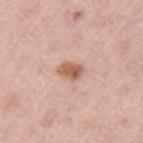Notes:
- follow-up · total-body-photography surveillance lesion; no biopsy
- lighting · white-light illumination
- patient · female, aged around 65
- location · the left upper arm
- imaging modality · total-body-photography crop, ~15 mm field of view
- image-analysis metrics · a footprint of about 4.5 mm², an eccentricity of roughly 0.75, and a symmetry-axis asymmetry near 0.25; an average lesion color of about L≈59 a*≈23 b*≈30 (CIELAB), a lesion–skin lightness drop of about 13, and a normalized lesion–skin contrast near 9; border irregularity of about 2 on a 0–10 scale and peripheral color asymmetry of about 1.5; a detector confidence of about 100 out of 100 that the crop contains a lesion